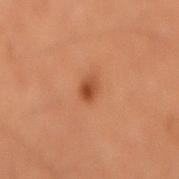{
  "biopsy_status": "not biopsied; imaged during a skin examination",
  "site": "lower back",
  "automated_metrics": {
    "area_mm2_approx": 3.0,
    "shape_asymmetry": 0.2,
    "nevus_likeness_0_100": 95,
    "lesion_detection_confidence_0_100": 100
  },
  "image": {
    "source": "total-body photography crop",
    "field_of_view_mm": 15
  },
  "lighting": "cross-polarized",
  "lesion_size": {
    "long_diameter_mm_approx": 2.5
  },
  "patient": {
    "sex": "male",
    "age_approx": 60
  }
}